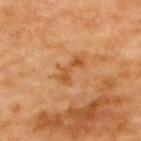biopsy_status: not biopsied; imaged during a skin examination
image:
  source: total-body photography crop
  field_of_view_mm: 15
lighting: cross-polarized
patient:
  age_approx: 65
lesion_size:
  long_diameter_mm_approx: 4.0
site: upper back